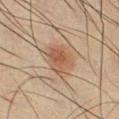Q: Was this lesion biopsied?
A: no biopsy performed (imaged during a skin exam)
Q: Where on the body is the lesion?
A: the chest
Q: What are the patient's age and sex?
A: male, in their mid- to late 30s
Q: What lighting was used for the tile?
A: cross-polarized illumination
Q: What did automated image analysis measure?
A: an automated nevus-likeness rating near 90 out of 100
Q: Lesion size?
A: ≈4.5 mm
Q: What kind of image is this?
A: 15 mm crop, total-body photography